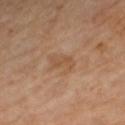biopsy status: total-body-photography surveillance lesion; no biopsy
patient: female, aged around 50
automated metrics: a lesion area of about 4 mm², an outline eccentricity of about 0.8 (0 = round, 1 = elongated), and a symmetry-axis asymmetry near 0.35; a border-irregularity index near 4/10 and radial color variation of about 0.5; a classifier nevus-likeness of about 0/100 and lesion-presence confidence of about 100/100
illumination: cross-polarized illumination
acquisition: 15 mm crop, total-body photography
body site: the right lower leg